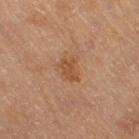Q: Was a biopsy performed?
A: total-body-photography surveillance lesion; no biopsy
Q: How was this image acquired?
A: 15 mm crop, total-body photography
Q: How large is the lesion?
A: ~3 mm (longest diameter)
Q: How was the tile lit?
A: cross-polarized
Q: What are the patient's age and sex?
A: female, aged approximately 55
Q: Automated lesion metrics?
A: a lesion color around L≈42 a*≈19 b*≈30 in CIELAB and a lesion–skin lightness drop of about 7
Q: Where on the body is the lesion?
A: the leg